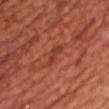Imaged during a routine full-body skin examination; the lesion was not biopsied and no histopathology is available. The lesion is located on the chest. A male patient, aged around 70. A 15 mm close-up extracted from a 3D total-body photography capture.